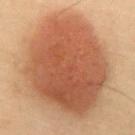Imaged during a routine full-body skin examination; the lesion was not biopsied and no histopathology is available.